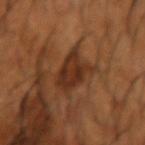This lesion was catalogued during total-body skin photography and was not selected for biopsy. A male subject, aged 63 to 67. Cropped from a total-body skin-imaging series; the visible field is about 15 mm. The lesion is located on the left forearm. Approximately 5.5 mm at its widest. This is a cross-polarized tile.